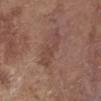Q: Was a biopsy performed?
A: imaged on a skin check; not biopsied
Q: Lesion location?
A: the arm
Q: What lighting was used for the tile?
A: white-light
Q: Who is the patient?
A: female, in their mid- to late 60s
Q: What is the imaging modality?
A: total-body-photography crop, ~15 mm field of view
Q: How large is the lesion?
A: ~5 mm (longest diameter)